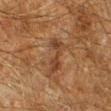Clinical summary: Longest diameter approximately 4.5 mm. On the right lower leg. A male patient, aged 58 to 62. An algorithmic analysis of the crop reported a lesion color around L≈31 a*≈17 b*≈26 in CIELAB, a lesion–skin lightness drop of about 7, and a normalized lesion–skin contrast near 7. It also reported a border-irregularity rating of about 6/10 and radial color variation of about 0.5. Imaged with cross-polarized lighting. Cropped from a total-body skin-imaging series; the visible field is about 15 mm.